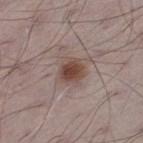Recorded during total-body skin imaging; not selected for excision or biopsy. A 15 mm close-up extracted from a 3D total-body photography capture. The patient is a male aged 68–72. Located on the left thigh. Imaged with white-light lighting.